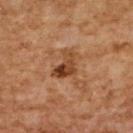<lesion>
<biopsy_status>not biopsied; imaged during a skin examination</biopsy_status>
<image>
  <source>total-body photography crop</source>
  <field_of_view_mm>15</field_of_view_mm>
</image>
<patient>
  <sex>female</sex>
  <age_approx>60</age_approx>
</patient>
<site>upper back</site>
</lesion>